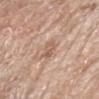Clinical impression:
This lesion was catalogued during total-body skin photography and was not selected for biopsy.
Acquisition and patient details:
A male subject aged 78 to 82. Approximately 2.5 mm at its widest. The lesion is on the arm. Cropped from a whole-body photographic skin survey; the tile spans about 15 mm. The total-body-photography lesion software estimated an area of roughly 3.5 mm² and an eccentricity of roughly 0.8. The software also gave a classifier nevus-likeness of about 0/100 and lesion-presence confidence of about 100/100. Imaged with white-light lighting.